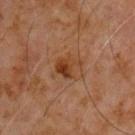| feature | finding |
|---|---|
| follow-up | imaged on a skin check; not biopsied |